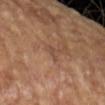The lesion was photographed on a routine skin check and not biopsied; there is no pathology result. Imaged with cross-polarized lighting. A roughly 15 mm field-of-view crop from a total-body skin photograph. The patient is a female aged around 70. On the left forearm. The lesion's longest dimension is about 2.5 mm.